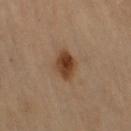The lesion was photographed on a routine skin check and not biopsied; there is no pathology result.